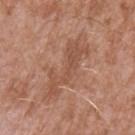biopsy status — imaged on a skin check; not biopsied | patient — male, aged 43 to 47 | location — the left upper arm | illumination — white-light illumination | automated lesion analysis — a border-irregularity index near 9/10, a color-variation rating of about 1.5/10, and a peripheral color-asymmetry measure near 0.5; a classifier nevus-likeness of about 0/100 | image — ~15 mm crop, total-body skin-cancer survey | lesion size — ≈7 mm.